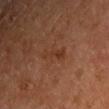{
  "biopsy_status": "not biopsied; imaged during a skin examination",
  "site": "right upper arm",
  "lighting": "cross-polarized",
  "lesion_size": {
    "long_diameter_mm_approx": 3.5
  },
  "image": {
    "source": "total-body photography crop",
    "field_of_view_mm": 15
  },
  "patient": {
    "sex": "male",
    "age_approx": 65
  }
}